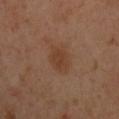<case>
<biopsy_status>not biopsied; imaged during a skin examination</biopsy_status>
<patient>
  <sex>male</sex>
  <age_approx>40</age_approx>
</patient>
<image>
  <source>total-body photography crop</source>
  <field_of_view_mm>15</field_of_view_mm>
</image>
<lighting>cross-polarized</lighting>
<site>chest</site>
<lesion_size>
  <long_diameter_mm_approx>4.0</long_diameter_mm_approx>
</lesion_size>
<automated_metrics>
  <cielab_L>39</cielab_L>
  <cielab_a>19</cielab_a>
  <cielab_b>29</cielab_b>
  <vs_skin_contrast_norm>6.0</vs_skin_contrast_norm>
  <border_irregularity_0_10>2.0</border_irregularity_0_10>
  <peripheral_color_asymmetry>0.5</peripheral_color_asymmetry>
</automated_metrics>
</case>